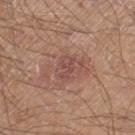* workup: imaged on a skin check; not biopsied
* site: the left thigh
* patient: male, aged approximately 20
* size: about 3 mm
* image source: ~15 mm crop, total-body skin-cancer survey